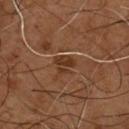Findings:
– workup — catalogued during a skin exam; not biopsied
– acquisition — total-body-photography crop, ~15 mm field of view
– site — the chest
– patient — male, about 55 years old